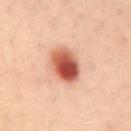Q: Was a biopsy performed?
A: total-body-photography surveillance lesion; no biopsy
Q: Lesion location?
A: the abdomen
Q: Automated lesion metrics?
A: a border-irregularity rating of about 1.5/10, a color-variation rating of about 9/10, and radial color variation of about 3; an automated nevus-likeness rating near 100 out of 100 and lesion-presence confidence of about 100/100
Q: Patient demographics?
A: male, roughly 40 years of age
Q: How was the tile lit?
A: cross-polarized illumination
Q: How large is the lesion?
A: ~4 mm (longest diameter)
Q: What is the imaging modality?
A: 15 mm crop, total-body photography